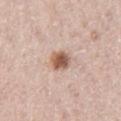* workup: imaged on a skin check; not biopsied
* patient: male, roughly 65 years of age
* location: the left thigh
* image: 15 mm crop, total-body photography
* lesion diameter: about 2.5 mm
* lighting: white-light